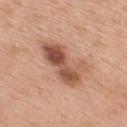Captured during whole-body skin photography for melanoma surveillance; the lesion was not biopsied.